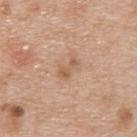{
  "biopsy_status": "not biopsied; imaged during a skin examination",
  "site": "upper back",
  "lighting": "white-light",
  "patient": {
    "sex": "male",
    "age_approx": 65
  },
  "image": {
    "source": "total-body photography crop",
    "field_of_view_mm": 15
  },
  "lesion_size": {
    "long_diameter_mm_approx": 2.5
  }
}